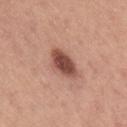  biopsy_status: not biopsied; imaged during a skin examination